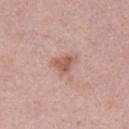{"biopsy_status": "not biopsied; imaged during a skin examination", "image": {"source": "total-body photography crop", "field_of_view_mm": 15}, "lesion_size": {"long_diameter_mm_approx": 3.0}, "lighting": "white-light", "automated_metrics": {"cielab_L": 56, "cielab_a": 25, "cielab_b": 25, "vs_skin_darker_L": 11.0, "vs_skin_contrast_norm": 7.5, "border_irregularity_0_10": 3.5, "color_variation_0_10": 2.5, "peripheral_color_asymmetry": 1.0}, "patient": {"sex": "male", "age_approx": 30}}